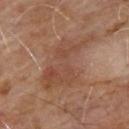* follow-up — no biopsy performed (imaged during a skin exam)
* lesion diameter — ≈8.5 mm
* subject — male, aged around 65
* imaging modality — 15 mm crop, total-body photography
* location — the upper back